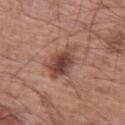Part of a total-body skin-imaging series; this lesion was reviewed on a skin check and was not flagged for biopsy. About 4.5 mm across. A roughly 15 mm field-of-view crop from a total-body skin photograph. The tile uses white-light illumination. The lesion is located on the right upper arm. A male patient, approximately 65 years of age.